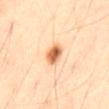Impression: The lesion was tiled from a total-body skin photograph and was not biopsied. Acquisition and patient details: The lesion-visualizer software estimated an average lesion color of about L≈67 a*≈25 b*≈41 (CIELAB), roughly 18 lightness units darker than nearby skin, and a lesion-to-skin contrast of about 10.5 (normalized; higher = more distinct). The analysis additionally found a color-variation rating of about 6/10 and a peripheral color-asymmetry measure near 2. It also reported a detector confidence of about 100 out of 100 that the crop contains a lesion. The recorded lesion diameter is about 3 mm. This is a cross-polarized tile. A male subject, roughly 55 years of age. A close-up tile cropped from a whole-body skin photograph, about 15 mm across. Located on the abdomen.